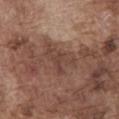biopsy_status: not biopsied; imaged during a skin examination
image:
  source: total-body photography crop
  field_of_view_mm: 15
patient:
  sex: male
  age_approx: 75
automated_metrics:
  area_mm2_approx: 8.0
  eccentricity: 0.75
  shape_asymmetry: 0.55
lighting: white-light
site: front of the torso
lesion_size:
  long_diameter_mm_approx: 4.5